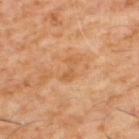Image and clinical context: Located on the upper back. The subject is a male aged around 60. Cropped from a total-body skin-imaging series; the visible field is about 15 mm.